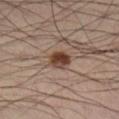Case summary:
• notes · total-body-photography surveillance lesion; no biopsy
• size · ≈2.5 mm
• lighting · cross-polarized
• patient · male, aged 38–42
• image-analysis metrics · a footprint of about 5.5 mm², an outline eccentricity of about 0.5 (0 = round, 1 = elongated), and a symmetry-axis asymmetry near 0.15; an automated nevus-likeness rating near 95 out of 100 and lesion-presence confidence of about 100/100
• image source · 15 mm crop, total-body photography
• body site · the right lower leg